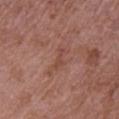Imaged during a routine full-body skin examination; the lesion was not biopsied and no histopathology is available. The patient is a female aged approximately 70. From the right upper arm. A close-up tile cropped from a whole-body skin photograph, about 15 mm across.